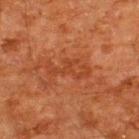This lesion was catalogued during total-body skin photography and was not selected for biopsy.
Automated image analysis of the tile measured border irregularity of about 8 on a 0–10 scale, a within-lesion color-variation index near 1.5/10, and a peripheral color-asymmetry measure near 0.5.
A roughly 15 mm field-of-view crop from a total-body skin photograph.
The subject is a male aged around 60.
Approximately 5 mm at its widest.
From the upper back.